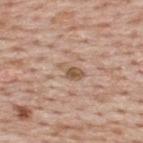Impression:
Captured during whole-body skin photography for melanoma surveillance; the lesion was not biopsied.
Acquisition and patient details:
The lesion is located on the upper back. A male patient in their mid-70s. Approximately 3 mm at its widest. The tile uses white-light illumination. A 15 mm close-up tile from a total-body photography series done for melanoma screening.